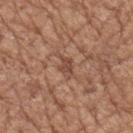| feature | finding |
|---|---|
| lesion diameter | about 2.5 mm |
| subject | female, aged 73–77 |
| body site | the arm |
| image source | 15 mm crop, total-body photography |
| lighting | white-light illumination |